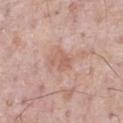Part of a total-body skin-imaging series; this lesion was reviewed on a skin check and was not flagged for biopsy.
A 15 mm close-up tile from a total-body photography series done for melanoma screening.
Imaged with white-light lighting.
The subject is a male aged around 70.
On the chest.